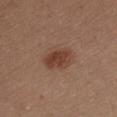Part of a total-body skin-imaging series; this lesion was reviewed on a skin check and was not flagged for biopsy.
From the chest.
A region of skin cropped from a whole-body photographic capture, roughly 15 mm wide.
A female patient, aged approximately 45.